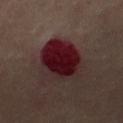No biopsy was performed on this lesion — it was imaged during a full skin examination and was not determined to be concerning.
From the leg.
A close-up tile cropped from a whole-body skin photograph, about 15 mm across.
A female patient in their 60s.
About 6 mm across.
Automated tile analysis of the lesion measured an average lesion color of about L≈20 a*≈24 b*≈16 (CIELAB) and a normalized border contrast of about 14.5.
This is a cross-polarized tile.